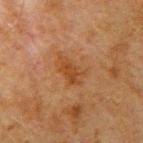Notes:
• follow-up: imaged on a skin check; not biopsied
• image source: 15 mm crop, total-body photography
• automated metrics: an area of roughly 6 mm², an outline eccentricity of about 0.85 (0 = round, 1 = elongated), and a symmetry-axis asymmetry near 0.3
• illumination: cross-polarized
• patient: male, approximately 80 years of age
• diameter: about 4 mm
• site: the arm